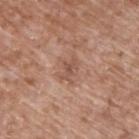Findings:
- workup · total-body-photography surveillance lesion; no biopsy
- tile lighting · white-light illumination
- site · the back
- size · ≈3 mm
- image · ~15 mm tile from a whole-body skin photo
- patient · male, in their mid- to late 60s